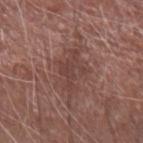Imaged during a routine full-body skin examination; the lesion was not biopsied and no histopathology is available.
Imaged with white-light lighting.
The lesion is on the right forearm.
The subject is a male roughly 65 years of age.
Automated tile analysis of the lesion measured a lesion area of about 8 mm², an eccentricity of roughly 0.85, and a shape-asymmetry score of about 0.45 (0 = symmetric). It also reported a lesion color around L≈41 a*≈20 b*≈22 in CIELAB and a lesion-to-skin contrast of about 6 (normalized; higher = more distinct). The software also gave a border-irregularity rating of about 8.5/10, internal color variation of about 2.5 on a 0–10 scale, and peripheral color asymmetry of about 1. And it measured a classifier nevus-likeness of about 0/100 and a lesion-detection confidence of about 95/100.
Measured at roughly 5 mm in maximum diameter.
A roughly 15 mm field-of-view crop from a total-body skin photograph.